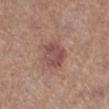Q: Was a biopsy performed?
A: imaged on a skin check; not biopsied
Q: What are the patient's age and sex?
A: female, aged 58 to 62
Q: Lesion location?
A: the right lower leg
Q: What is the imaging modality?
A: ~15 mm crop, total-body skin-cancer survey